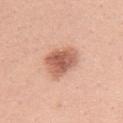No biopsy was performed on this lesion — it was imaged during a full skin examination and was not determined to be concerning.
On the back.
Cropped from a whole-body photographic skin survey; the tile spans about 15 mm.
A female subject approximately 40 years of age.
Longest diameter approximately 4.5 mm.